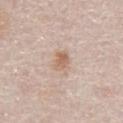Context:
Automated tile analysis of the lesion measured a shape eccentricity near 0.7 and a symmetry-axis asymmetry near 0.2. And it measured a lesion–skin lightness drop of about 9. A female patient aged approximately 60. A roughly 15 mm field-of-view crop from a total-body skin photograph. The lesion is located on the abdomen.